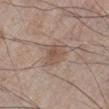Captured during whole-body skin photography for melanoma surveillance; the lesion was not biopsied. From the left lower leg. A male subject, aged approximately 70. A region of skin cropped from a whole-body photographic capture, roughly 15 mm wide. Imaged with white-light lighting.